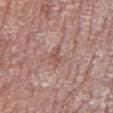Case summary:
– biopsy status · total-body-photography surveillance lesion; no biopsy
– patient · male, aged around 60
– image · total-body-photography crop, ~15 mm field of view
– site · the leg
– TBP lesion metrics · a lesion area of about 2.5 mm², an eccentricity of roughly 0.85, and a shape-asymmetry score of about 0.55 (0 = symmetric); lesion-presence confidence of about 100/100
– tile lighting · white-light
– diameter · ~2.5 mm (longest diameter)